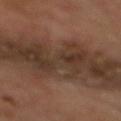Notes:
– workup — imaged on a skin check; not biopsied
– image — 15 mm crop, total-body photography
– patient — male, in their 70s
– site — the mid back
– lighting — cross-polarized illumination
– TBP lesion metrics — a lesion area of about 125 mm², an eccentricity of roughly 0.95, and two-axis asymmetry of about 0.25; border irregularity of about 6 on a 0–10 scale and a peripheral color-asymmetry measure near 2; a nevus-likeness score of about 0/100 and a lesion-detection confidence of about 65/100